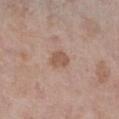Clinical impression: The lesion was tiled from a total-body skin photograph and was not biopsied. Acquisition and patient details: The lesion is on the left lower leg. A 15 mm crop from a total-body photograph taken for skin-cancer surveillance. Longest diameter approximately 2.5 mm. A male patient in their 70s. Imaged with white-light lighting.